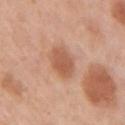Q: Was this lesion biopsied?
A: no biopsy performed (imaged during a skin exam)
Q: Automated lesion metrics?
A: an area of roughly 8 mm² and a shape-asymmetry score of about 0.15 (0 = symmetric); a border-irregularity rating of about 1.5/10, a within-lesion color-variation index near 2/10, and peripheral color asymmetry of about 0.5
Q: Where on the body is the lesion?
A: the arm
Q: How was this image acquired?
A: ~15 mm tile from a whole-body skin photo
Q: How large is the lesion?
A: ~3 mm (longest diameter)
Q: What are the patient's age and sex?
A: female, about 45 years old